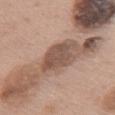Assessment:
This lesion was catalogued during total-body skin photography and was not selected for biopsy.
Clinical summary:
The patient is a female aged approximately 60. Cropped from a total-body skin-imaging series; the visible field is about 15 mm. The lesion is on the front of the torso. The tile uses white-light illumination. Longest diameter approximately 4 mm.